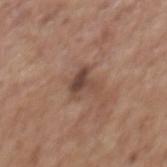Background: The subject is a female roughly 65 years of age. Cropped from a whole-body photographic skin survey; the tile spans about 15 mm. The lesion is located on the mid back. Measured at roughly 3 mm in maximum diameter. Automated image analysis of the tile measured a footprint of about 6 mm², an outline eccentricity of about 0.45 (0 = round, 1 = elongated), and two-axis asymmetry of about 0.45. The software also gave a classifier nevus-likeness of about 10/100 and lesion-presence confidence of about 100/100.